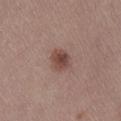site: the back; lesion diameter: ≈3 mm; patient: female, approximately 50 years of age; image source: ~15 mm tile from a whole-body skin photo.